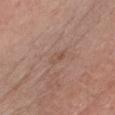Impression: The lesion was photographed on a routine skin check and not biopsied; there is no pathology result. Context: A 15 mm crop from a total-body photograph taken for skin-cancer surveillance. Automated image analysis of the tile measured a classifier nevus-likeness of about 0/100 and a lesion-detection confidence of about 100/100. A female subject, in their 40s. Imaged with white-light lighting. From the chest. Approximately 2.5 mm at its widest.